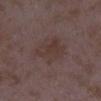Q: Was this lesion biopsied?
A: catalogued during a skin exam; not biopsied
Q: What did automated image analysis measure?
A: an automated nevus-likeness rating near 0 out of 100
Q: How large is the lesion?
A: about 4.5 mm
Q: Who is the patient?
A: female, approximately 35 years of age
Q: What is the imaging modality?
A: 15 mm crop, total-body photography
Q: How was the tile lit?
A: white-light
Q: Where on the body is the lesion?
A: the leg